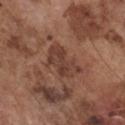notes = no biopsy performed (imaged during a skin exam) | imaging modality = ~15 mm tile from a whole-body skin photo | subject = male, roughly 75 years of age | anatomic site = the chest.